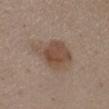The lesion was photographed on a routine skin check and not biopsied; there is no pathology result. On the chest. About 5 mm across. A region of skin cropped from a whole-body photographic capture, roughly 15 mm wide. Captured under white-light illumination. A female patient, roughly 35 years of age.